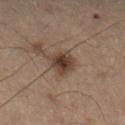Q: Is there a histopathology result?
A: imaged on a skin check; not biopsied
Q: What is the imaging modality?
A: ~15 mm crop, total-body skin-cancer survey
Q: Patient demographics?
A: male, aged around 55
Q: How large is the lesion?
A: ~3.5 mm (longest diameter)
Q: Lesion location?
A: the right lower leg